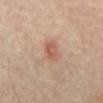Case summary:
- biopsy status: total-body-photography surveillance lesion; no biopsy